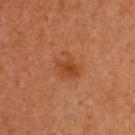Assessment:
The lesion was photographed on a routine skin check and not biopsied; there is no pathology result.
Clinical summary:
The subject is a female aged 48–52. This is a cross-polarized tile. The total-body-photography lesion software estimated a classifier nevus-likeness of about 65/100 and lesion-presence confidence of about 100/100. A lesion tile, about 15 mm wide, cut from a 3D total-body photograph. About 3.5 mm across.